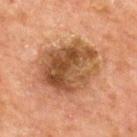Captured during whole-body skin photography for melanoma surveillance; the lesion was not biopsied.
A male patient, aged approximately 65.
The lesion is located on the chest.
The total-body-photography lesion software estimated border irregularity of about 2 on a 0–10 scale, a within-lesion color-variation index near 8/10, and peripheral color asymmetry of about 3. It also reported a detector confidence of about 100 out of 100 that the crop contains a lesion.
The recorded lesion diameter is about 7.5 mm.
A lesion tile, about 15 mm wide, cut from a 3D total-body photograph.
Imaged with cross-polarized lighting.